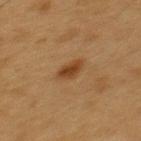Assessment:
The lesion was tiled from a total-body skin photograph and was not biopsied.
Image and clinical context:
A close-up tile cropped from a whole-body skin photograph, about 15 mm across. The recorded lesion diameter is about 3 mm. The subject is a male in their mid-50s. From the upper back.